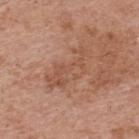{
  "biopsy_status": "not biopsied; imaged during a skin examination",
  "site": "upper back",
  "lighting": "white-light",
  "image": {
    "source": "total-body photography crop",
    "field_of_view_mm": 15
  },
  "patient": {
    "sex": "male",
    "age_approx": 70
  }
}